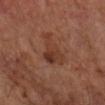Clinical impression:
Part of a total-body skin-imaging series; this lesion was reviewed on a skin check and was not flagged for biopsy.
Acquisition and patient details:
This is a cross-polarized tile. Cropped from a total-body skin-imaging series; the visible field is about 15 mm. Located on the right forearm. The lesion-visualizer software estimated an average lesion color of about L≈32 a*≈19 b*≈25 (CIELAB), about 7 CIELAB-L* units darker than the surrounding skin, and a lesion-to-skin contrast of about 6.5 (normalized; higher = more distinct). The patient is a male aged 63–67. About 5 mm across.